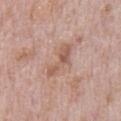The lesion was photographed on a routine skin check and not biopsied; there is no pathology result. Cropped from a total-body skin-imaging series; the visible field is about 15 mm. The lesion is on the chest. The patient is a male aged 68–72. Measured at roughly 5 mm in maximum diameter. Captured under white-light illumination.